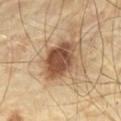The patient is a male aged 68–72.
The tile uses cross-polarized illumination.
About 5.5 mm across.
The lesion is located on the chest.
Cropped from a total-body skin-imaging series; the visible field is about 15 mm.
The total-body-photography lesion software estimated roughly 16 lightness units darker than nearby skin and a normalized lesion–skin contrast near 10.5. The software also gave a border-irregularity rating of about 2/10 and peripheral color asymmetry of about 1.5.